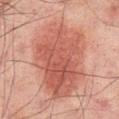Part of a total-body skin-imaging series; this lesion was reviewed on a skin check and was not flagged for biopsy. A male patient, in their mid- to late 60s. The total-body-photography lesion software estimated an average lesion color of about L≈44 a*≈23 b*≈24 (CIELAB), about 9 CIELAB-L* units darker than the surrounding skin, and a lesion-to-skin contrast of about 7 (normalized; higher = more distinct). From the back. Measured at roughly 9.5 mm in maximum diameter. The tile uses cross-polarized illumination. A region of skin cropped from a whole-body photographic capture, roughly 15 mm wide.